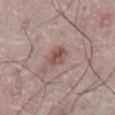biopsy status: imaged on a skin check; not biopsied | patient: male, in their mid-70s | location: the abdomen | imaging modality: ~15 mm crop, total-body skin-cancer survey.